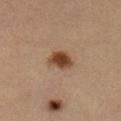notes: total-body-photography surveillance lesion; no biopsy | lighting: cross-polarized illumination | acquisition: 15 mm crop, total-body photography | patient: female, aged around 30 | site: the right lower leg.